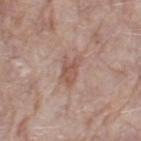Impression:
Recorded during total-body skin imaging; not selected for excision or biopsy.
Acquisition and patient details:
This is a white-light tile. The subject is a female aged approximately 70. A lesion tile, about 15 mm wide, cut from a 3D total-body photograph. Located on the left thigh.